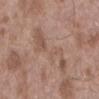This lesion was catalogued during total-body skin photography and was not selected for biopsy. Located on the lower back. A male patient in their mid-50s. Automated tile analysis of the lesion measured a shape eccentricity near 0.95. The analysis additionally found a mean CIELAB color near L≈54 a*≈18 b*≈26, a lesion–skin lightness drop of about 6, and a lesion-to-skin contrast of about 5 (normalized; higher = more distinct). The tile uses white-light illumination. A 15 mm close-up extracted from a 3D total-body photography capture.